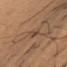Imaged during a routine full-body skin examination; the lesion was not biopsied and no histopathology is available. Cropped from a whole-body photographic skin survey; the tile spans about 15 mm. The lesion is located on the chest. Approximately 3 mm at its widest. The subject is a male in their mid-50s. Automated image analysis of the tile measured a lesion area of about 2.5 mm² and a shape-asymmetry score of about 0.4 (0 = symmetric). It also reported a mean CIELAB color near L≈43 a*≈17 b*≈28, about 6 CIELAB-L* units darker than the surrounding skin, and a normalized lesion–skin contrast near 5.5. And it measured a border-irregularity index near 4.5/10 and radial color variation of about 0. And it measured a classifier nevus-likeness of about 10/100.